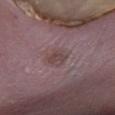Impression: No biopsy was performed on this lesion — it was imaged during a full skin examination and was not determined to be concerning. Background: A region of skin cropped from a whole-body photographic capture, roughly 15 mm wide. Automated image analysis of the tile measured an average lesion color of about L≈43 a*≈17 b*≈17 (CIELAB) and roughly 7 lightness units darker than nearby skin. It also reported a border-irregularity rating of about 2.5/10, a within-lesion color-variation index near 3/10, and radial color variation of about 1. From the right lower leg. The lesion's longest dimension is about 3 mm. The tile uses white-light illumination. The subject is a male aged 58 to 62.